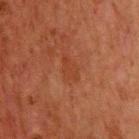Notes:
* biopsy status: no biopsy performed (imaged during a skin exam)
* tile lighting: cross-polarized
* patient: male, about 65 years old
* imaging modality: ~15 mm tile from a whole-body skin photo
* diameter: ~3 mm (longest diameter)
* body site: the chest